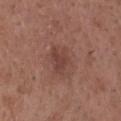Clinical impression: The lesion was photographed on a routine skin check and not biopsied; there is no pathology result. Clinical summary: A male patient, aged around 35. Imaged with white-light lighting. This image is a 15 mm lesion crop taken from a total-body photograph. The lesion is on the head or neck.